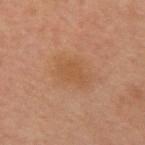Case summary:
- biopsy status: imaged on a skin check; not biopsied
- lighting: cross-polarized
- patient: male, about 60 years old
- acquisition: 15 mm crop, total-body photography
- automated metrics: border irregularity of about 3.5 on a 0–10 scale, a within-lesion color-variation index near 1.5/10, and peripheral color asymmetry of about 0.5
- site: the front of the torso
- lesion size: about 3.5 mm